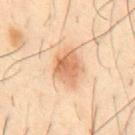| feature | finding |
|---|---|
| notes | total-body-photography surveillance lesion; no biopsy |
| anatomic site | the abdomen |
| diameter | ≈4 mm |
| patient | male, about 40 years old |
| tile lighting | cross-polarized illumination |
| acquisition | ~15 mm tile from a whole-body skin photo |